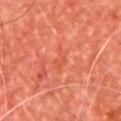follow-up: total-body-photography surveillance lesion; no biopsy.